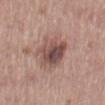Cropped from a total-body skin-imaging series; the visible field is about 15 mm. The subject is a female about 65 years old. The lesion is located on the mid back. Captured under white-light illumination. The total-body-photography lesion software estimated a shape-asymmetry score of about 0.2 (0 = symmetric). It also reported a lesion color around L≈49 a*≈19 b*≈22 in CIELAB, a lesion–skin lightness drop of about 13, and a normalized border contrast of about 9. The analysis additionally found internal color variation of about 6.5 on a 0–10 scale and peripheral color asymmetry of about 2. The software also gave a classifier nevus-likeness of about 35/100 and lesion-presence confidence of about 100/100. The recorded lesion diameter is about 4 mm.